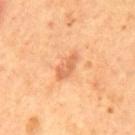biopsy status: catalogued during a skin exam; not biopsied | patient: male, roughly 55 years of age | imaging modality: 15 mm crop, total-body photography | tile lighting: cross-polarized illumination | lesion diameter: ~3.5 mm (longest diameter) | location: the mid back.